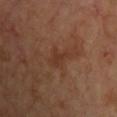Notes:
– biopsy status — catalogued during a skin exam; not biopsied
– site — the chest
– tile lighting — cross-polarized
– lesion diameter — ≈2.5 mm
– imaging modality — ~15 mm tile from a whole-body skin photo
– patient — aged around 65